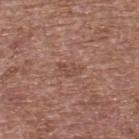The lesion was photographed on a routine skin check and not biopsied; there is no pathology result.
A 15 mm close-up tile from a total-body photography series done for melanoma screening.
From the upper back.
A female patient, aged approximately 65.
Imaged with white-light lighting.
Measured at roughly 2.5 mm in maximum diameter.